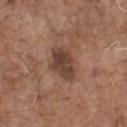Assessment: Imaged during a routine full-body skin examination; the lesion was not biopsied and no histopathology is available. Context: The lesion is on the front of the torso. The subject is a male aged 68–72. A roughly 15 mm field-of-view crop from a total-body skin photograph.